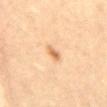{"patient": {"sex": "male", "age_approx": 20}, "image": {"source": "total-body photography crop", "field_of_view_mm": 15}, "site": "abdomen", "lesion_size": {"long_diameter_mm_approx": 2.5}}